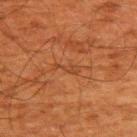No biopsy was performed on this lesion — it was imaged during a full skin examination and was not determined to be concerning.
Measured at roughly 3 mm in maximum diameter.
The lesion-visualizer software estimated a shape eccentricity near 0.95 and a shape-asymmetry score of about 0.5 (0 = symmetric). It also reported a normalized lesion–skin contrast near 5. And it measured a border-irregularity rating of about 6/10, a color-variation rating of about 0/10, and peripheral color asymmetry of about 0.
This is a cross-polarized tile.
This image is a 15 mm lesion crop taken from a total-body photograph.
From the back.
The patient is a male aged around 60.